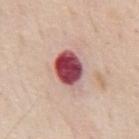Assessment: Recorded during total-body skin imaging; not selected for excision or biopsy.